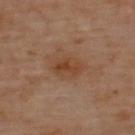biopsy status: no biopsy performed (imaged during a skin exam); acquisition: ~15 mm crop, total-body skin-cancer survey; site: the back; lighting: cross-polarized illumination; lesion diameter: ~3 mm (longest diameter).